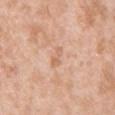TBP lesion metrics: an area of roughly 3.5 mm², an eccentricity of roughly 0.85, and a shape-asymmetry score of about 0.4 (0 = symmetric); an average lesion color of about L≈65 a*≈21 b*≈33 (CIELAB) and a normalized border contrast of about 4.5; a within-lesion color-variation index near 1.5/10 and a peripheral color-asymmetry measure near 0.5; a classifier nevus-likeness of about 0/100 and lesion-presence confidence of about 100/100 | lesion diameter: ~2.5 mm (longest diameter) | anatomic site: the chest | subject: male, aged 38–42 | lighting: white-light illumination | image: 15 mm crop, total-body photography.